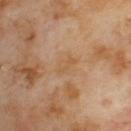workup: imaged on a skin check; not biopsied | diameter: ~2.5 mm (longest diameter) | imaging modality: ~15 mm crop, total-body skin-cancer survey | anatomic site: the upper back | tile lighting: cross-polarized illumination | TBP lesion metrics: an average lesion color of about L≈54 a*≈18 b*≈36 (CIELAB) and a normalized lesion–skin contrast near 5 | patient: male, roughly 70 years of age.